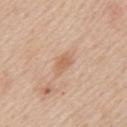No biopsy was performed on this lesion — it was imaged during a full skin examination and was not determined to be concerning. Cropped from a whole-body photographic skin survey; the tile spans about 15 mm. On the upper back. A male subject, in their 60s. Measured at roughly 3 mm in maximum diameter.